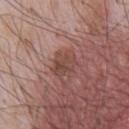Q: Was this lesion biopsied?
A: catalogued during a skin exam; not biopsied
Q: How was this image acquired?
A: ~15 mm crop, total-body skin-cancer survey
Q: Illumination type?
A: white-light illumination
Q: Lesion size?
A: ~3.5 mm (longest diameter)
Q: What are the patient's age and sex?
A: male, roughly 65 years of age
Q: Where on the body is the lesion?
A: the front of the torso
Q: Automated lesion metrics?
A: a footprint of about 6.5 mm², an outline eccentricity of about 0.65 (0 = round, 1 = elongated), and a symmetry-axis asymmetry near 0.3; a lesion-to-skin contrast of about 6 (normalized; higher = more distinct); a classifier nevus-likeness of about 0/100 and lesion-presence confidence of about 100/100